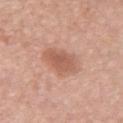{"site": "chest", "lighting": "white-light", "image": {"source": "total-body photography crop", "field_of_view_mm": 15}, "patient": {"sex": "female", "age_approx": 60}, "lesion_size": {"long_diameter_mm_approx": 4.5}, "automated_metrics": {"area_mm2_approx": 10.0, "cielab_L": 58, "cielab_a": 23, "cielab_b": 30, "vs_skin_contrast_norm": 6.5, "border_irregularity_0_10": 2.5, "color_variation_0_10": 2.5, "peripheral_color_asymmetry": 1.0, "lesion_detection_confidence_0_100": 100}}